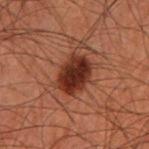biopsy_status: not biopsied; imaged during a skin examination
image:
  source: total-body photography crop
  field_of_view_mm: 15
patient:
  sex: male
  age_approx: 60
automated_metrics:
  area_mm2_approx: 12.0
  eccentricity: 0.7
  shape_asymmetry: 0.15
  cielab_L: 23
  cielab_a: 21
  cielab_b: 23
  vs_skin_contrast_norm: 12.5
  color_variation_0_10: 4.5
  peripheral_color_asymmetry: 1.0
lighting: cross-polarized
site: right forearm